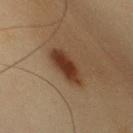illumination: cross-polarized illumination; lesion diameter: ~6.5 mm (longest diameter); subject: male, in their mid-30s; image source: 15 mm crop, total-body photography; site: the chest.